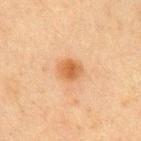workup: imaged on a skin check; not biopsied
body site: the abdomen
tile lighting: cross-polarized
acquisition: total-body-photography crop, ~15 mm field of view
size: ~2.5 mm (longest diameter)
image-analysis metrics: roughly 9 lightness units darker than nearby skin; border irregularity of about 1.5 on a 0–10 scale, a color-variation rating of about 3/10, and peripheral color asymmetry of about 1
patient: male, aged approximately 75